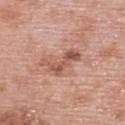Imaged during a routine full-body skin examination; the lesion was not biopsied and no histopathology is available. A female patient, aged 58 to 62. The tile uses white-light illumination. A 15 mm crop from a total-body photograph taken for skin-cancer surveillance. Longest diameter approximately 4.5 mm. Located on the upper back.